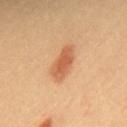Recorded during total-body skin imaging; not selected for excision or biopsy. A female subject aged 28–32. The tile uses cross-polarized illumination. The lesion is located on the upper back. The total-body-photography lesion software estimated a footprint of about 7.5 mm² and a symmetry-axis asymmetry near 0.2. The software also gave roughly 12 lightness units darker than nearby skin. The software also gave a classifier nevus-likeness of about 100/100 and a lesion-detection confidence of about 100/100. Longest diameter approximately 4.5 mm. A 15 mm close-up extracted from a 3D total-body photography capture.